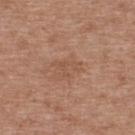Imaged during a routine full-body skin examination; the lesion was not biopsied and no histopathology is available.
A close-up tile cropped from a whole-body skin photograph, about 15 mm across.
The lesion is located on the upper back.
The recorded lesion diameter is about 3.5 mm.
A female patient aged approximately 40.
Imaged with white-light lighting.